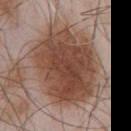| key | value |
|---|---|
| workup | catalogued during a skin exam; not biopsied |
| size | about 10 mm |
| patient | male, aged 53–57 |
| image source | ~15 mm crop, total-body skin-cancer survey |
| location | the chest |